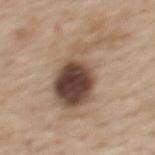Part of a total-body skin-imaging series; this lesion was reviewed on a skin check and was not flagged for biopsy. Located on the upper back. A female patient, aged around 50. A roughly 15 mm field-of-view crop from a total-body skin photograph.